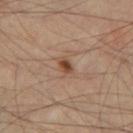* biopsy status — catalogued during a skin exam; not biopsied
* automated lesion analysis — an automated nevus-likeness rating near 95 out of 100
* location — the leg
* tile lighting — cross-polarized
* subject — male, aged 53–57
* imaging modality — total-body-photography crop, ~15 mm field of view
* lesion size — ~2 mm (longest diameter)